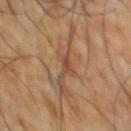No biopsy was performed on this lesion — it was imaged during a full skin examination and was not determined to be concerning. A male subject roughly 65 years of age. The lesion-visualizer software estimated a footprint of about 2.5 mm². And it measured an average lesion color of about L≈44 a*≈19 b*≈29 (CIELAB), about 8 CIELAB-L* units darker than the surrounding skin, and a lesion-to-skin contrast of about 6.5 (normalized; higher = more distinct). It also reported a border-irregularity index near 5/10, internal color variation of about 0 on a 0–10 scale, and peripheral color asymmetry of about 0. The analysis additionally found a nevus-likeness score of about 0/100 and a lesion-detection confidence of about 55/100. A 15 mm close-up extracted from a 3D total-body photography capture. The lesion is on the chest.